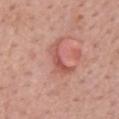Assessment: No biopsy was performed on this lesion — it was imaged during a full skin examination and was not determined to be concerning. Context: The lesion is on the back. A 15 mm close-up tile from a total-body photography series done for melanoma screening. Captured under white-light illumination. A female patient, aged around 65. The recorded lesion diameter is about 4.5 mm.